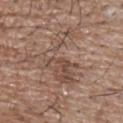Context: The lesion's longest dimension is about 7 mm. A male patient in their mid- to late 60s. The lesion-visualizer software estimated an area of roughly 15 mm², an outline eccentricity of about 0.8 (0 = round, 1 = elongated), and a shape-asymmetry score of about 0.75 (0 = symmetric). Captured under white-light illumination. From the upper back. A 15 mm close-up tile from a total-body photography series done for melanoma screening.